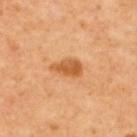Assessment: Part of a total-body skin-imaging series; this lesion was reviewed on a skin check and was not flagged for biopsy. Acquisition and patient details: The patient is a female in their 50s. Located on the upper back. An algorithmic analysis of the crop reported a footprint of about 5.5 mm², an eccentricity of roughly 0.85, and two-axis asymmetry of about 0.3. And it measured an average lesion color of about L≈60 a*≈28 b*≈45 (CIELAB), roughly 12 lightness units darker than nearby skin, and a normalized lesion–skin contrast near 8. A 15 mm close-up tile from a total-body photography series done for melanoma screening.